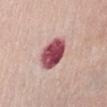A 15 mm close-up extracted from a 3D total-body photography capture.
Imaged with white-light lighting.
The lesion's longest dimension is about 4 mm.
The lesion is located on the abdomen.
The subject is a female in their mid-60s.